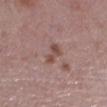<case>
  <biopsy_status>not biopsied; imaged during a skin examination</biopsy_status>
  <image>
    <source>total-body photography crop</source>
    <field_of_view_mm>15</field_of_view_mm>
  </image>
  <patient>
    <sex>female</sex>
    <age_approx>65</age_approx>
  </patient>
  <lesion_size>
    <long_diameter_mm_approx>3.0</long_diameter_mm_approx>
  </lesion_size>
  <site>left lower leg</site>
  <automated_metrics>
    <nevus_likeness_0_100>5</nevus_likeness_0_100>
    <lesion_detection_confidence_0_100>100</lesion_detection_confidence_0_100>
  </automated_metrics>
</case>